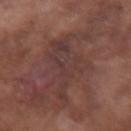Imaged during a routine full-body skin examination; the lesion was not biopsied and no histopathology is available. This image is a 15 mm lesion crop taken from a total-body photograph. From the arm. A male subject aged around 75.